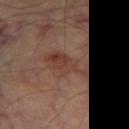The lesion was photographed on a routine skin check and not biopsied; there is no pathology result. Longest diameter approximately 5.5 mm. On the left thigh. A 15 mm close-up extracted from a 3D total-body photography capture. A male subject, about 70 years old.